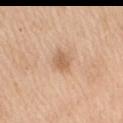The lesion was photographed on a routine skin check and not biopsied; there is no pathology result. The lesion is on the right upper arm. The patient is a female roughly 55 years of age. A roughly 15 mm field-of-view crop from a total-body skin photograph. Imaged with white-light lighting. About 2.5 mm across.